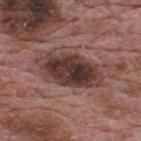| key | value |
|---|---|
| biopsy status | total-body-photography surveillance lesion; no biopsy |
| diameter | ~8 mm (longest diameter) |
| tile lighting | white-light illumination |
| subject | male, approximately 70 years of age |
| body site | the upper back |
| acquisition | ~15 mm tile from a whole-body skin photo |